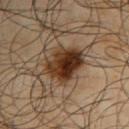This lesion was catalogued during total-body skin photography and was not selected for biopsy.
Cropped from a whole-body photographic skin survey; the tile spans about 15 mm.
This is a cross-polarized tile.
On the right upper arm.
A male patient aged around 65.
Measured at roughly 5 mm in maximum diameter.